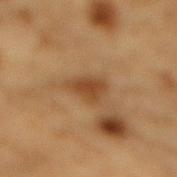- workup · imaged on a skin check; not biopsied
- imaging modality · 15 mm crop, total-body photography
- patient · male, aged around 85 or older
- location · the mid back